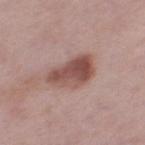Clinical impression: Recorded during total-body skin imaging; not selected for excision or biopsy. Context: The subject is a female aged around 40. Captured under white-light illumination. From the left thigh. About 5 mm across. This image is a 15 mm lesion crop taken from a total-body photograph.